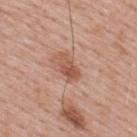Notes:
– workup — catalogued during a skin exam; not biopsied
– lesion size — ≈3.5 mm
– tile lighting — white-light
– patient — male, aged around 40
– imaging modality — 15 mm crop, total-body photography
– TBP lesion metrics — a lesion area of about 5.5 mm², an outline eccentricity of about 0.85 (0 = round, 1 = elongated), and two-axis asymmetry of about 0.25; a border-irregularity rating of about 3/10; a detector confidence of about 100 out of 100 that the crop contains a lesion
– location — the upper back